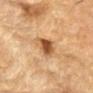Recorded during total-body skin imaging; not selected for excision or biopsy.
This image is a 15 mm lesion crop taken from a total-body photograph.
The lesion is on the arm.
A male subject aged approximately 85 or older.